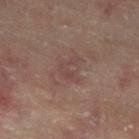Clinical impression: Part of a total-body skin-imaging series; this lesion was reviewed on a skin check and was not flagged for biopsy. Image and clinical context: Imaged with cross-polarized lighting. Approximately 3 mm at its widest. The patient is a male roughly 60 years of age. A region of skin cropped from a whole-body photographic capture, roughly 15 mm wide. On the left lower leg.